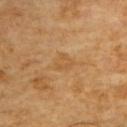Q: Was a biopsy performed?
A: imaged on a skin check; not biopsied
Q: What lighting was used for the tile?
A: cross-polarized
Q: What is the lesion's diameter?
A: ≈2.5 mm
Q: What is the anatomic site?
A: the upper back
Q: Who is the patient?
A: male, in their 60s
Q: What kind of image is this?
A: ~15 mm tile from a whole-body skin photo